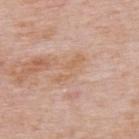The lesion was tiled from a total-body skin photograph and was not biopsied. Cropped from a whole-body photographic skin survey; the tile spans about 15 mm. Longest diameter approximately 4.5 mm. A male subject, roughly 75 years of age. Imaged with white-light lighting. The lesion is on the back.